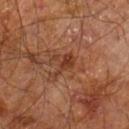Recorded during total-body skin imaging; not selected for excision or biopsy. Longest diameter approximately 3.5 mm. On the leg. A close-up tile cropped from a whole-body skin photograph, about 15 mm across. A male patient about 60 years old. Captured under cross-polarized illumination.